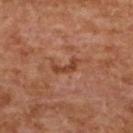| field | value |
|---|---|
| biopsy status | catalogued during a skin exam; not biopsied |
| illumination | cross-polarized illumination |
| image source | 15 mm crop, total-body photography |
| body site | the upper back |
| diameter | ~3.5 mm (longest diameter) |
| subject | female, aged approximately 60 |